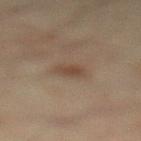Impression:
Part of a total-body skin-imaging series; this lesion was reviewed on a skin check and was not flagged for biopsy.
Image and clinical context:
A 15 mm close-up extracted from a 3D total-body photography capture. Captured under cross-polarized illumination. On the left lower leg. The patient is a male aged around 45.